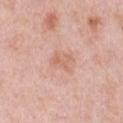Case summary:
- follow-up: imaged on a skin check; not biopsied
- body site: the chest
- image source: ~15 mm crop, total-body skin-cancer survey
- lighting: white-light
- patient: female, about 65 years old